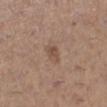<case>
<site>left lower leg</site>
<image>
  <source>total-body photography crop</source>
  <field_of_view_mm>15</field_of_view_mm>
</image>
<patient>
  <sex>female</sex>
  <age_approx>45</age_approx>
</patient>
<lesion_size>
  <long_diameter_mm_approx>2.5</long_diameter_mm_approx>
</lesion_size>
<lighting>white-light</lighting>
</case>